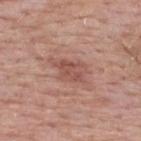Case summary:
* notes · catalogued during a skin exam; not biopsied
* lesion size · about 4.5 mm
* image · ~15 mm crop, total-body skin-cancer survey
* image-analysis metrics · a nevus-likeness score of about 5/100 and a lesion-detection confidence of about 100/100
* patient · male, aged approximately 55
* illumination · white-light
* body site · the upper back